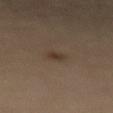No biopsy was performed on this lesion — it was imaged during a full skin examination and was not determined to be concerning. A lesion tile, about 15 mm wide, cut from a 3D total-body photograph. Longest diameter approximately 2 mm. Imaged with cross-polarized lighting. The patient is a female in their mid-60s. Located on the abdomen.